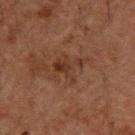This lesion was catalogued during total-body skin photography and was not selected for biopsy. The subject is a male aged around 50. The lesion is located on the upper back. This image is a 15 mm lesion crop taken from a total-body photograph.